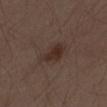<tbp_lesion>
  <biopsy_status>not biopsied; imaged during a skin examination</biopsy_status>
  <image>
    <source>total-body photography crop</source>
    <field_of_view_mm>15</field_of_view_mm>
  </image>
  <patient>
    <sex>male</sex>
    <age_approx>70</age_approx>
  </patient>
  <site>abdomen</site>
</tbp_lesion>